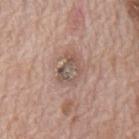{"biopsy_status": "not biopsied; imaged during a skin examination", "automated_metrics": {"cielab_L": 53, "cielab_a": 15, "cielab_b": 23, "vs_skin_darker_L": 9.0, "vs_skin_contrast_norm": 6.5, "border_irregularity_0_10": 4.0, "color_variation_0_10": 5.0, "peripheral_color_asymmetry": 1.5}, "image": {"source": "total-body photography crop", "field_of_view_mm": 15}, "site": "mid back", "patient": {"sex": "male", "age_approx": 70}, "lighting": "white-light", "lesion_size": {"long_diameter_mm_approx": 3.5}}